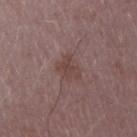The lesion was photographed on a routine skin check and not biopsied; there is no pathology result.
Longest diameter approximately 3 mm.
A region of skin cropped from a whole-body photographic capture, roughly 15 mm wide.
The patient is a female aged around 45.
The lesion is on the right upper arm.
This is a white-light tile.
An algorithmic analysis of the crop reported a footprint of about 5 mm², a shape eccentricity near 0.75, and two-axis asymmetry of about 0.45. The analysis additionally found a lesion color around L≈42 a*≈19 b*≈20 in CIELAB, about 7 CIELAB-L* units darker than the surrounding skin, and a lesion-to-skin contrast of about 6.5 (normalized; higher = more distinct).